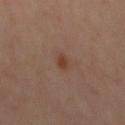This lesion was catalogued during total-body skin photography and was not selected for biopsy.
On the mid back.
A lesion tile, about 15 mm wide, cut from a 3D total-body photograph.
The tile uses cross-polarized illumination.
The lesion-visualizer software estimated a footprint of about 3 mm² and an eccentricity of roughly 0.9. The software also gave border irregularity of about 3 on a 0–10 scale and internal color variation of about 1 on a 0–10 scale.
A male subject roughly 65 years of age.